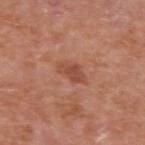Notes:
– patient — male, in their mid-60s
– site — the upper back
– image source — total-body-photography crop, ~15 mm field of view
– illumination — white-light illumination
– size — about 3 mm